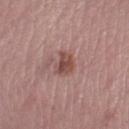Q: Where on the body is the lesion?
A: the leg
Q: Patient demographics?
A: female, aged 43 to 47
Q: How was this image acquired?
A: ~15 mm tile from a whole-body skin photo